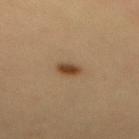Impression:
The lesion was photographed on a routine skin check and not biopsied; there is no pathology result.
Context:
Measured at roughly 2.5 mm in maximum diameter. The tile uses cross-polarized illumination. A close-up tile cropped from a whole-body skin photograph, about 15 mm across. From the upper back. A female subject aged 28–32.